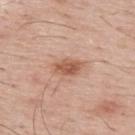Q: What is the imaging modality?
A: ~15 mm crop, total-body skin-cancer survey
Q: Who is the patient?
A: male, roughly 65 years of age
Q: What is the anatomic site?
A: the upper back